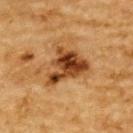Q: Was this lesion biopsied?
A: imaged on a skin check; not biopsied
Q: What kind of image is this?
A: 15 mm crop, total-body photography
Q: Lesion location?
A: the upper back
Q: Who is the patient?
A: male, aged approximately 85 or older
Q: How large is the lesion?
A: about 5.5 mm
Q: How was the tile lit?
A: cross-polarized illumination